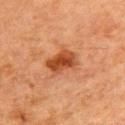site: chest
image:
  source: total-body photography crop
  field_of_view_mm: 15
patient:
  sex: male
  age_approx: 80